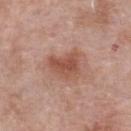<case>
<biopsy_status>not biopsied; imaged during a skin examination</biopsy_status>
<lighting>white-light</lighting>
<image>
  <source>total-body photography crop</source>
  <field_of_view_mm>15</field_of_view_mm>
</image>
<automated_metrics>
  <shape_asymmetry>0.4</shape_asymmetry>
  <nevus_likeness_0_100>55</nevus_likeness_0_100>
</automated_metrics>
<site>front of the torso</site>
<lesion_size>
  <long_diameter_mm_approx>4.0</long_diameter_mm_approx>
</lesion_size>
<patient>
  <sex>male</sex>
  <age_approx>55</age_approx>
</patient>
</case>